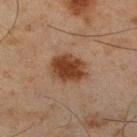Recorded during total-body skin imaging; not selected for excision or biopsy.
A male subject approximately 45 years of age.
The total-body-photography lesion software estimated an area of roughly 12 mm² and a shape eccentricity near 0.65. The analysis additionally found a border-irregularity index near 1.5/10 and a within-lesion color-variation index near 3/10. The analysis additionally found an automated nevus-likeness rating near 100 out of 100 and a detector confidence of about 100 out of 100 that the crop contains a lesion.
Measured at roughly 4.5 mm in maximum diameter.
A region of skin cropped from a whole-body photographic capture, roughly 15 mm wide.
The tile uses cross-polarized illumination.
From the leg.